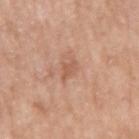The lesion was tiled from a total-body skin photograph and was not biopsied. This image is a 15 mm lesion crop taken from a total-body photograph. From the right upper arm. About 3 mm across. The lesion-visualizer software estimated an outline eccentricity of about 0.85 (0 = round, 1 = elongated) and two-axis asymmetry of about 0.35. It also reported a mean CIELAB color near L≈57 a*≈23 b*≈31 and a lesion-to-skin contrast of about 6 (normalized; higher = more distinct). The analysis additionally found a border-irregularity rating of about 4/10, a within-lesion color-variation index near 0.5/10, and a peripheral color-asymmetry measure near 0. The analysis additionally found a classifier nevus-likeness of about 0/100 and a lesion-detection confidence of about 100/100. A male patient roughly 65 years of age. This is a white-light tile.